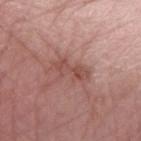lesion size = ~4.5 mm (longest diameter) | image source = total-body-photography crop, ~15 mm field of view | location = the arm | illumination = white-light | subject = female, approximately 60 years of age.